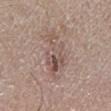biopsy status = total-body-photography surveillance lesion; no biopsy | subject = male, roughly 50 years of age | location = the leg | lighting = white-light illumination | lesion size = ≈6.5 mm | automated metrics = an average lesion color of about L≈53 a*≈16 b*≈22 (CIELAB), roughly 9 lightness units darker than nearby skin, and a normalized lesion–skin contrast near 6; border irregularity of about 7.5 on a 0–10 scale, internal color variation of about 9.5 on a 0–10 scale, and radial color variation of about 3 | acquisition = 15 mm crop, total-body photography.